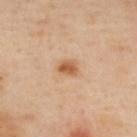Case summary:
- workup · no biopsy performed (imaged during a skin exam)
- imaging modality · ~15 mm tile from a whole-body skin photo
- patient · female, in their 40s
- anatomic site · the upper back